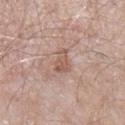| feature | finding |
|---|---|
| workup | no biopsy performed (imaged during a skin exam) |
| subject | male, aged 63–67 |
| image | total-body-photography crop, ~15 mm field of view |
| anatomic site | the right upper arm |